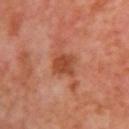No biopsy was performed on this lesion — it was imaged during a full skin examination and was not determined to be concerning.
Imaged with cross-polarized lighting.
Measured at roughly 3.5 mm in maximum diameter.
A female patient approximately 50 years of age.
On the right upper arm.
This image is a 15 mm lesion crop taken from a total-body photograph.